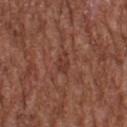Clinical impression: Captured during whole-body skin photography for melanoma surveillance; the lesion was not biopsied. Context: The total-body-photography lesion software estimated about 6 CIELAB-L* units darker than the surrounding skin and a normalized lesion–skin contrast near 5.5. The software also gave a border-irregularity rating of about 3/10 and radial color variation of about 1. The analysis additionally found a lesion-detection confidence of about 90/100. The lesion is on the upper back. Cropped from a total-body skin-imaging series; the visible field is about 15 mm. A male patient, aged around 75.